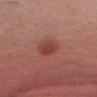The total-body-photography lesion software estimated a footprint of about 7 mm², a shape eccentricity near 0.6, and a symmetry-axis asymmetry near 0.1. And it measured a border-irregularity index near 1/10, internal color variation of about 3.5 on a 0–10 scale, and peripheral color asymmetry of about 1. The analysis additionally found a classifier nevus-likeness of about 70/100 and a detector confidence of about 100 out of 100 that the crop contains a lesion. On the head or neck. A roughly 15 mm field-of-view crop from a total-body skin photograph. A male patient, approximately 35 years of age. Captured under white-light illumination. Approximately 3 mm at its widest.